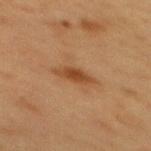image = 15 mm crop, total-body photography | subject = female, aged 48 to 52 | diameter = ~3.5 mm (longest diameter) | site = the mid back | illumination = cross-polarized illumination.